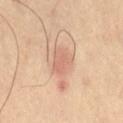Impression:
Imaged during a routine full-body skin examination; the lesion was not biopsied and no histopathology is available.
Image and clinical context:
The lesion's longest dimension is about 3.5 mm. Imaged with cross-polarized lighting. A 15 mm close-up tile from a total-body photography series done for melanoma screening. On the abdomen.